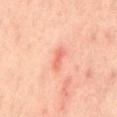Recorded during total-body skin imaging; not selected for excision or biopsy. A 15 mm close-up extracted from a 3D total-body photography capture. On the mid back. A female subject, aged 38 to 42.